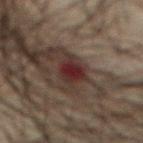illumination: cross-polarized
lesion diameter: ≈4 mm
patient: male, about 65 years old
image-analysis metrics: a footprint of about 6 mm², a shape eccentricity near 0.8, and two-axis asymmetry of about 0.25; internal color variation of about 4 on a 0–10 scale and a peripheral color-asymmetry measure near 1; a classifier nevus-likeness of about 0/100 and lesion-presence confidence of about 100/100
site: the abdomen
imaging modality: 15 mm crop, total-body photography